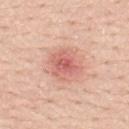Case summary:
* follow-up — no biopsy performed (imaged during a skin exam)
* lesion size — about 4 mm
* location — the mid back
* illumination — white-light illumination
* patient — male, aged approximately 50
* image-analysis metrics — roughly 11 lightness units darker than nearby skin and a lesion-to-skin contrast of about 6.5 (normalized; higher = more distinct); lesion-presence confidence of about 100/100
* imaging modality — ~15 mm tile from a whole-body skin photo